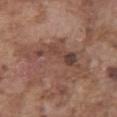- notes — total-body-photography surveillance lesion; no biopsy
- patient — male, aged 73–77
- acquisition — 15 mm crop, total-body photography
- body site — the abdomen
- illumination — white-light illumination
- image-analysis metrics — an area of roughly 22 mm², a shape eccentricity near 0.9, and a symmetry-axis asymmetry near 0.6; a lesion color around L≈45 a*≈19 b*≈25 in CIELAB and a lesion-to-skin contrast of about 6.5 (normalized; higher = more distinct); border irregularity of about 9.5 on a 0–10 scale, a color-variation rating of about 6.5/10, and radial color variation of about 2
- diameter — ≈9.5 mm